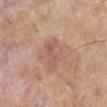notes — imaged on a skin check; not biopsied
location — the left lower leg
imaging modality — ~15 mm crop, total-body skin-cancer survey
subject — male, roughly 65 years of age
size — ≈4.5 mm